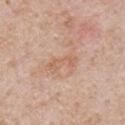Longest diameter approximately 4 mm. The lesion is on the chest. A region of skin cropped from a whole-body photographic capture, roughly 15 mm wide. The lesion-visualizer software estimated an area of roughly 4.5 mm² and an eccentricity of roughly 0.95. It also reported a border-irregularity index near 5/10, a within-lesion color-variation index near 1/10, and a peripheral color-asymmetry measure near 0. And it measured a nevus-likeness score of about 0/100 and a detector confidence of about 100 out of 100 that the crop contains a lesion. A male patient, in their 70s.